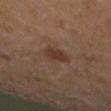Q: Was a biopsy performed?
A: total-body-photography surveillance lesion; no biopsy
Q: Who is the patient?
A: male, aged 63–67
Q: What is the imaging modality?
A: total-body-photography crop, ~15 mm field of view
Q: What did automated image analysis measure?
A: an average lesion color of about L≈32 a*≈17 b*≈24 (CIELAB) and a lesion-to-skin contrast of about 7.5 (normalized; higher = more distinct)
Q: Lesion location?
A: the mid back
Q: Lesion size?
A: ~2.5 mm (longest diameter)
Q: What lighting was used for the tile?
A: cross-polarized